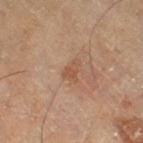subject: male, aged 63–67 | image-analysis metrics: an average lesion color of about L≈53 a*≈20 b*≈32 (CIELAB) and a lesion–skin lightness drop of about 7 | image: ~15 mm crop, total-body skin-cancer survey | diameter: ~3 mm (longest diameter) | lighting: cross-polarized illumination | location: the right thigh.